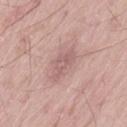{"biopsy_status": "not biopsied; imaged during a skin examination", "lesion_size": {"long_diameter_mm_approx": 4.5}, "image": {"source": "total-body photography crop", "field_of_view_mm": 15}, "patient": {"sex": "male", "age_approx": 60}, "site": "lower back", "lighting": "white-light"}